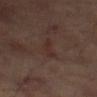notes — catalogued during a skin exam; not biopsied
lesion diameter — ≈3.5 mm
body site — the left thigh
acquisition — total-body-photography crop, ~15 mm field of view
automated metrics — an area of roughly 3.5 mm², a shape eccentricity near 0.95, and a symmetry-axis asymmetry near 0.5; a lesion color around L≈27 a*≈17 b*≈19 in CIELAB and a normalized border contrast of about 5.5
lighting — cross-polarized
subject — male, approximately 70 years of age